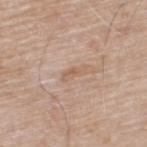Q: Was a biopsy performed?
A: catalogued during a skin exam; not biopsied
Q: Automated lesion metrics?
A: a footprint of about 2.5 mm² and two-axis asymmetry of about 0.45; a lesion–skin lightness drop of about 7 and a lesion-to-skin contrast of about 5.5 (normalized; higher = more distinct); a detector confidence of about 100 out of 100 that the crop contains a lesion
Q: Illumination type?
A: white-light illumination
Q: What is the imaging modality?
A: ~15 mm tile from a whole-body skin photo
Q: Where on the body is the lesion?
A: the upper back
Q: What are the patient's age and sex?
A: male, aged around 65
Q: What is the lesion's diameter?
A: about 3 mm